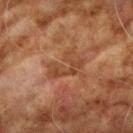Q: Was this lesion biopsied?
A: imaged on a skin check; not biopsied
Q: What lighting was used for the tile?
A: cross-polarized
Q: What did automated image analysis measure?
A: an average lesion color of about L≈36 a*≈20 b*≈29 (CIELAB), a lesion–skin lightness drop of about 6, and a normalized lesion–skin contrast near 6; a border-irregularity rating of about 7.5/10, a color-variation rating of about 2.5/10, and a peripheral color-asymmetry measure near 1
Q: Where on the body is the lesion?
A: the arm
Q: Who is the patient?
A: male, in their 70s
Q: How large is the lesion?
A: ~4.5 mm (longest diameter)
Q: What is the imaging modality?
A: ~15 mm crop, total-body skin-cancer survey